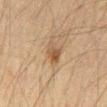The patient is a male aged 58 to 62.
The lesion is on the chest.
A close-up tile cropped from a whole-body skin photograph, about 15 mm across.
The lesion-visualizer software estimated a footprint of about 4.5 mm², an outline eccentricity of about 0.75 (0 = round, 1 = elongated), and two-axis asymmetry of about 0.25. The analysis additionally found an average lesion color of about L≈40 a*≈14 b*≈27 (CIELAB) and a lesion–skin lightness drop of about 8.